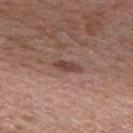Assessment:
No biopsy was performed on this lesion — it was imaged during a full skin examination and was not determined to be concerning.
Context:
The patient is a male aged 63–67. Located on the mid back. The lesion's longest dimension is about 3 mm. An algorithmic analysis of the crop reported an eccentricity of roughly 0.9 and a shape-asymmetry score of about 0.3 (0 = symmetric). It also reported a border-irregularity rating of about 3/10, a color-variation rating of about 1.5/10, and peripheral color asymmetry of about 0. The analysis additionally found an automated nevus-likeness rating near 15 out of 100. A 15 mm close-up extracted from a 3D total-body photography capture. The tile uses white-light illumination.